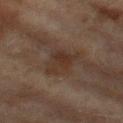biopsy status = no biopsy performed (imaged during a skin exam); site = the left thigh; subject = female, aged approximately 60; image = total-body-photography crop, ~15 mm field of view.